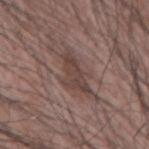Clinical impression: Captured during whole-body skin photography for melanoma surveillance; the lesion was not biopsied. Background: On the left forearm. This is a white-light tile. Cropped from a whole-body photographic skin survey; the tile spans about 15 mm. The subject is a male aged around 75.